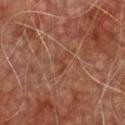Recorded during total-body skin imaging; not selected for excision or biopsy.
Automated image analysis of the tile measured a border-irregularity index near 8/10 and a color-variation rating of about 0/10. The analysis additionally found an automated nevus-likeness rating near 0 out of 100 and a detector confidence of about 85 out of 100 that the crop contains a lesion.
The tile uses cross-polarized illumination.
The patient is a male in their mid- to late 70s.
The lesion is located on the front of the torso.
A 15 mm crop from a total-body photograph taken for skin-cancer surveillance.